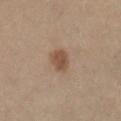No biopsy was performed on this lesion — it was imaged during a full skin examination and was not determined to be concerning.
The patient is a female aged 48 to 52.
The lesion's longest dimension is about 2.5 mm.
This is a cross-polarized tile.
A 15 mm close-up extracted from a 3D total-body photography capture.
The lesion is on the left lower leg.